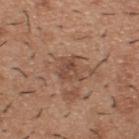Q: Is there a histopathology result?
A: total-body-photography surveillance lesion; no biopsy
Q: What lighting was used for the tile?
A: white-light
Q: What is the lesion's diameter?
A: ≈3 mm
Q: Patient demographics?
A: male, aged around 40
Q: Lesion location?
A: the upper back
Q: What is the imaging modality?
A: ~15 mm crop, total-body skin-cancer survey